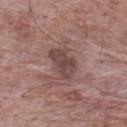Impression:
The lesion was tiled from a total-body skin photograph and was not biopsied.
Clinical summary:
A roughly 15 mm field-of-view crop from a total-body skin photograph. A male subject aged 68–72. Captured under white-light illumination. From the back. Measured at roughly 4 mm in maximum diameter.